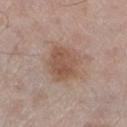  biopsy_status: not biopsied; imaged during a skin examination
  site: left lower leg
  lighting: white-light
  patient:
    sex: male
    age_approx: 60
  lesion_size:
    long_diameter_mm_approx: 4.5
  image:
    source: total-body photography crop
    field_of_view_mm: 15